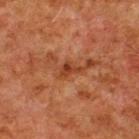  automated_metrics:
    border_irregularity_0_10: 5.0
    color_variation_0_10: 2.0
    peripheral_color_asymmetry: 0.5
    nevus_likeness_0_100: 0
  site: back
  lighting: cross-polarized
  lesion_size:
    long_diameter_mm_approx: 2.5
  patient:
    sex: male
    age_approx: 80
  image:
    source: total-body photography crop
    field_of_view_mm: 15